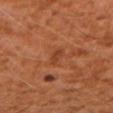Case summary:
• anatomic site — the right upper arm
• image-analysis metrics — a shape eccentricity near 0.95 and a symmetry-axis asymmetry near 0.2; a lesion color around L≈38 a*≈26 b*≈33 in CIELAB, about 7 CIELAB-L* units darker than the surrounding skin, and a normalized border contrast of about 6.5
• lighting — cross-polarized illumination
• lesion diameter — ≈2.5 mm
• subject — male, in their 60s
• imaging modality — 15 mm crop, total-body photography